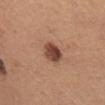Impression:
This lesion was catalogued during total-body skin photography and was not selected for biopsy.
Acquisition and patient details:
A female patient, roughly 65 years of age. Approximately 3 mm at its widest. A 15 mm close-up tile from a total-body photography series done for melanoma screening. Captured under white-light illumination. On the chest.